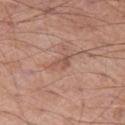This lesion was catalogued during total-body skin photography and was not selected for biopsy.
A 15 mm close-up extracted from a 3D total-body photography capture.
The lesion's longest dimension is about 2.5 mm.
This is a white-light tile.
A male patient aged around 55.
Located on the left thigh.